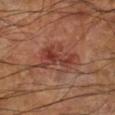The lesion was photographed on a routine skin check and not biopsied; there is no pathology result.
A region of skin cropped from a whole-body photographic capture, roughly 15 mm wide.
A male patient, in their 60s.
From the left lower leg.